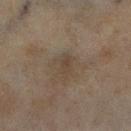biopsy status — imaged on a skin check; not biopsied
patient — female, about 60 years old
lesion diameter — ≈3 mm
tile lighting — cross-polarized illumination
image source — ~15 mm crop, total-body skin-cancer survey
automated metrics — a footprint of about 5 mm², an outline eccentricity of about 0.7 (0 = round, 1 = elongated), and two-axis asymmetry of about 0.3; a mean CIELAB color near L≈38 a*≈10 b*≈23, a lesion–skin lightness drop of about 5, and a normalized border contrast of about 5; border irregularity of about 3 on a 0–10 scale and peripheral color asymmetry of about 1; a nevus-likeness score of about 0/100
location — the right lower leg